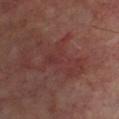Clinical impression:
This lesion was catalogued during total-body skin photography and was not selected for biopsy.
Background:
The lesion-visualizer software estimated a footprint of about 16 mm² and an eccentricity of roughly 0.8. The analysis additionally found a lesion color around L≈34 a*≈23 b*≈21 in CIELAB and roughly 5 lightness units darker than nearby skin. The software also gave a nevus-likeness score of about 0/100 and a lesion-detection confidence of about 95/100. A subject aged 53 to 57. This is a cross-polarized tile. From the chest. Cropped from a whole-body photographic skin survey; the tile spans about 15 mm.